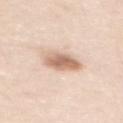Q: Lesion size?
A: ~3.5 mm (longest diameter)
Q: What kind of image is this?
A: ~15 mm crop, total-body skin-cancer survey
Q: How was the tile lit?
A: white-light illumination
Q: Patient demographics?
A: female, aged 48 to 52
Q: Automated lesion metrics?
A: an average lesion color of about L≈67 a*≈19 b*≈30 (CIELAB) and a normalized border contrast of about 8.5
Q: Where on the body is the lesion?
A: the back